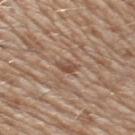Part of a total-body skin-imaging series; this lesion was reviewed on a skin check and was not flagged for biopsy.
On the chest.
The lesion's longest dimension is about 2.5 mm.
The tile uses white-light illumination.
An algorithmic analysis of the crop reported a footprint of about 2.5 mm², an eccentricity of roughly 0.85, and a shape-asymmetry score of about 0.3 (0 = symmetric).
The patient is a male in their 60s.
A 15 mm close-up tile from a total-body photography series done for melanoma screening.